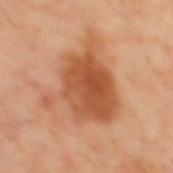Clinical impression: Part of a total-body skin-imaging series; this lesion was reviewed on a skin check and was not flagged for biopsy. Background: This is a cross-polarized tile. A 15 mm close-up extracted from a 3D total-body photography capture. The patient is a male in their 60s. The lesion is on the mid back. The lesion-visualizer software estimated an eccentricity of roughly 0.6.